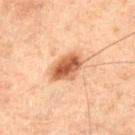follow-up: total-body-photography surveillance lesion; no biopsy
lesion size: about 4 mm
acquisition: ~15 mm crop, total-body skin-cancer survey
anatomic site: the abdomen
tile lighting: cross-polarized illumination
automated lesion analysis: border irregularity of about 1.5 on a 0–10 scale, internal color variation of about 5.5 on a 0–10 scale, and a peripheral color-asymmetry measure near 1.5; a classifier nevus-likeness of about 100/100 and a detector confidence of about 100 out of 100 that the crop contains a lesion
patient: male, about 70 years old